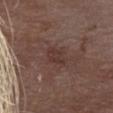acquisition — total-body-photography crop, ~15 mm field of view
automated lesion analysis — border irregularity of about 3 on a 0–10 scale and a color-variation rating of about 1.5/10; a classifier nevus-likeness of about 0/100
subject — female, aged 78–82
tile lighting — white-light illumination
body site — the head or neck
size — about 3 mm